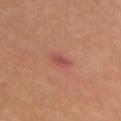workup = total-body-photography surveillance lesion; no biopsy | site = the chest | image = 15 mm crop, total-body photography | subject = female, aged approximately 55 | lesion size = ~2.5 mm (longest diameter).